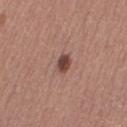Findings:
* tile lighting: white-light illumination
* image source: total-body-photography crop, ~15 mm field of view
* site: the right thigh
* TBP lesion metrics: an automated nevus-likeness rating near 95 out of 100 and a detector confidence of about 100 out of 100 that the crop contains a lesion
* patient: female, aged 38 to 42
* diameter: ≈2 mm